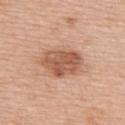No biopsy was performed on this lesion — it was imaged during a full skin examination and was not determined to be concerning.